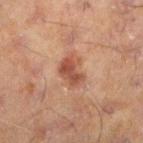A close-up tile cropped from a whole-body skin photograph, about 15 mm across. The recorded lesion diameter is about 3.5 mm. Automated tile analysis of the lesion measured a lesion color around L≈38 a*≈20 b*≈24 in CIELAB, about 9 CIELAB-L* units darker than the surrounding skin, and a normalized border contrast of about 7.5. The software also gave radial color variation of about 1. The analysis additionally found a classifier nevus-likeness of about 30/100 and a lesion-detection confidence of about 100/100. A male patient approximately 60 years of age. Imaged with cross-polarized lighting. From the leg.